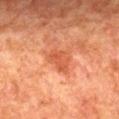Assessment:
Part of a total-body skin-imaging series; this lesion was reviewed on a skin check and was not flagged for biopsy.
Acquisition and patient details:
A male patient approximately 80 years of age. A 15 mm close-up tile from a total-body photography series done for melanoma screening. On the mid back. Longest diameter approximately 3.5 mm. An algorithmic analysis of the crop reported a mean CIELAB color near L≈44 a*≈27 b*≈33 and a normalized lesion–skin contrast near 6.5. The software also gave a border-irregularity index near 3.5/10, a color-variation rating of about 2.5/10, and peripheral color asymmetry of about 1. The analysis additionally found a classifier nevus-likeness of about 0/100 and lesion-presence confidence of about 100/100. Imaged with cross-polarized lighting.